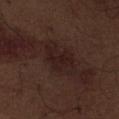biopsy status = no biopsy performed (imaged during a skin exam); acquisition = 15 mm crop, total-body photography; patient = male, about 70 years old; location = the abdomen.